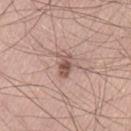Impression:
The lesion was tiled from a total-body skin photograph and was not biopsied.
Background:
The total-body-photography lesion software estimated a lesion color around L≈53 a*≈20 b*≈25 in CIELAB and a lesion-to-skin contrast of about 8 (normalized; higher = more distinct). The analysis additionally found a border-irregularity index near 2/10, internal color variation of about 3.5 on a 0–10 scale, and radial color variation of about 1. Located on the lower back. Imaged with white-light lighting. The subject is a male aged approximately 60. Approximately 2.5 mm at its widest. A close-up tile cropped from a whole-body skin photograph, about 15 mm across.